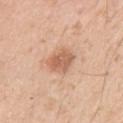Longest diameter approximately 3 mm. Imaged with white-light lighting. A male patient roughly 55 years of age. The lesion is located on the right upper arm. A close-up tile cropped from a whole-body skin photograph, about 15 mm across.